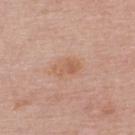Imaged during a routine full-body skin examination; the lesion was not biopsied and no histopathology is available. The lesion-visualizer software estimated an outline eccentricity of about 0.85 (0 = round, 1 = elongated). It also reported roughly 7 lightness units darker than nearby skin. And it measured a border-irregularity index near 3.5/10, a color-variation rating of about 2/10, and a peripheral color-asymmetry measure near 0.5. A female subject approximately 50 years of age. This image is a 15 mm lesion crop taken from a total-body photograph. Longest diameter approximately 3 mm. Imaged with white-light lighting. On the upper back.